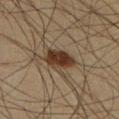Impression:
This lesion was catalogued during total-body skin photography and was not selected for biopsy.